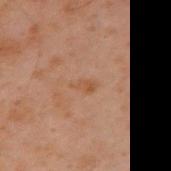Clinical impression:
The lesion was tiled from a total-body skin photograph and was not biopsied.
Context:
A male patient about 50 years old. The lesion is on the arm. The lesion-visualizer software estimated a lesion area of about 2.5 mm², an outline eccentricity of about 0.85 (0 = round, 1 = elongated), and a symmetry-axis asymmetry near 0.3. The software also gave a lesion color around L≈42 a*≈17 b*≈29 in CIELAB, about 5 CIELAB-L* units darker than the surrounding skin, and a lesion-to-skin contrast of about 5 (normalized; higher = more distinct). The software also gave a border-irregularity index near 3.5/10, a within-lesion color-variation index near 1.5/10, and a peripheral color-asymmetry measure near 0.5. Imaged with cross-polarized lighting. Cropped from a whole-body photographic skin survey; the tile spans about 15 mm. The lesion's longest dimension is about 2.5 mm.